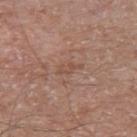- diameter · ~3 mm (longest diameter)
- image-analysis metrics · a peripheral color-asymmetry measure near 0
- image source · total-body-photography crop, ~15 mm field of view
- subject · male, roughly 65 years of age
- location · the left upper arm
- lighting · white-light illumination